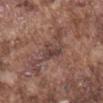No biopsy was performed on this lesion — it was imaged during a full skin examination and was not determined to be concerning.
On the mid back.
Automated image analysis of the tile measured a lesion area of about 6.5 mm², an outline eccentricity of about 0.7 (0 = round, 1 = elongated), and two-axis asymmetry of about 0.35. The analysis additionally found about 8 CIELAB-L* units darker than the surrounding skin and a normalized lesion–skin contrast near 6.5. It also reported border irregularity of about 4 on a 0–10 scale, a within-lesion color-variation index near 4/10, and a peripheral color-asymmetry measure near 1.5. And it measured a classifier nevus-likeness of about 0/100 and a lesion-detection confidence of about 50/100.
A region of skin cropped from a whole-body photographic capture, roughly 15 mm wide.
A male patient aged approximately 75.
Captured under white-light illumination.